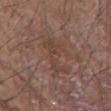follow-up: catalogued during a skin exam; not biopsied | subject: male, in their 80s | tile lighting: white-light illumination | TBP lesion metrics: a mean CIELAB color near L≈42 a*≈17 b*≈24, about 5 CIELAB-L* units darker than the surrounding skin, and a lesion-to-skin contrast of about 5 (normalized; higher = more distinct); border irregularity of about 9.5 on a 0–10 scale and peripheral color asymmetry of about 0.5 | location: the arm | lesion diameter: ≈5 mm | imaging modality: ~15 mm crop, total-body skin-cancer survey.